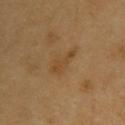Q: Was a biopsy performed?
A: catalogued during a skin exam; not biopsied
Q: What are the patient's age and sex?
A: male, aged 58–62
Q: Lesion size?
A: ~3.5 mm (longest diameter)
Q: Where on the body is the lesion?
A: the upper back
Q: What is the imaging modality?
A: ~15 mm crop, total-body skin-cancer survey